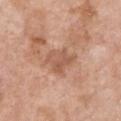No biopsy was performed on this lesion — it was imaged during a full skin examination and was not determined to be concerning.
A female subject aged approximately 75.
A 15 mm close-up extracted from a 3D total-body photography capture.
Longest diameter approximately 3.5 mm.
On the chest.